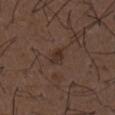Clinical impression:
The lesion was photographed on a routine skin check and not biopsied; there is no pathology result.
Clinical summary:
The recorded lesion diameter is about 2.5 mm. Automated image analysis of the tile measured about 6 CIELAB-L* units darker than the surrounding skin and a normalized lesion–skin contrast near 6.5. And it measured a within-lesion color-variation index near 2.5/10. And it measured a nevus-likeness score of about 5/100 and a detector confidence of about 100 out of 100 that the crop contains a lesion. This image is a 15 mm lesion crop taken from a total-body photograph. Imaged with white-light lighting. A male patient aged 48 to 52. The lesion is located on the chest.